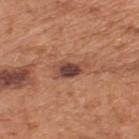Q: Is there a histopathology result?
A: no biopsy performed (imaged during a skin exam)
Q: Lesion location?
A: the upper back
Q: What are the patient's age and sex?
A: male, approximately 65 years of age
Q: What kind of image is this?
A: total-body-photography crop, ~15 mm field of view